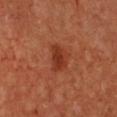On the chest. A 15 mm close-up tile from a total-body photography series done for melanoma screening. A female subject, in their mid- to late 40s.